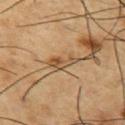<tbp_lesion>
  <biopsy_status>not biopsied; imaged during a skin examination</biopsy_status>
  <image>
    <source>total-body photography crop</source>
    <field_of_view_mm>15</field_of_view_mm>
  </image>
  <site>chest</site>
  <lighting>cross-polarized</lighting>
  <lesion_size>
    <long_diameter_mm_approx>3.5</long_diameter_mm_approx>
  </lesion_size>
  <automated_metrics>
    <nevus_likeness_0_100>0</nevus_likeness_0_100>
  </automated_metrics>
  <patient>
    <sex>male</sex>
    <age_approx>55</age_approx>
  </patient>
</tbp_lesion>